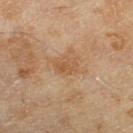image: ~15 mm tile from a whole-body skin photo | subject: female, approximately 60 years of age | anatomic site: the right lower leg.